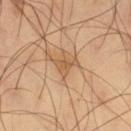Q: Was this lesion biopsied?
A: catalogued during a skin exam; not biopsied
Q: What are the patient's age and sex?
A: male, approximately 60 years of age
Q: How large is the lesion?
A: ≈3 mm
Q: What is the anatomic site?
A: the right thigh
Q: What is the imaging modality?
A: ~15 mm tile from a whole-body skin photo
Q: How was the tile lit?
A: cross-polarized